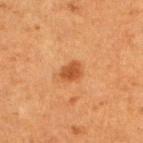Assessment: Imaged during a routine full-body skin examination; the lesion was not biopsied and no histopathology is available. Acquisition and patient details: Longest diameter approximately 2.5 mm. The tile uses cross-polarized illumination. Automated image analysis of the tile measured a nevus-likeness score of about 95/100. From the left lower leg. The subject is a female aged 48 to 52. A 15 mm close-up extracted from a 3D total-body photography capture.